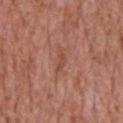{
  "biopsy_status": "not biopsied; imaged during a skin examination",
  "lighting": "white-light",
  "patient": {
    "sex": "male",
    "age_approx": 65
  },
  "site": "chest",
  "automated_metrics": {
    "area_mm2_approx": 2.0,
    "eccentricity": 0.95,
    "shape_asymmetry": 0.4,
    "color_variation_0_10": 0.0,
    "peripheral_color_asymmetry": 0.0
  },
  "lesion_size": {
    "long_diameter_mm_approx": 2.5
  },
  "image": {
    "source": "total-body photography crop",
    "field_of_view_mm": 15
  }
}